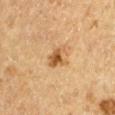Recorded during total-body skin imaging; not selected for excision or biopsy. A close-up tile cropped from a whole-body skin photograph, about 15 mm across. A male patient, aged 63–67. The lesion is on the right upper arm. The lesion-visualizer software estimated an eccentricity of roughly 0.55 and a symmetry-axis asymmetry near 0.3. The lesion's longest dimension is about 2.5 mm.